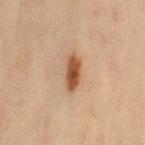Notes:
* biopsy status: catalogued during a skin exam; not biopsied
* image: ~15 mm tile from a whole-body skin photo
* patient: female, approximately 45 years of age
* site: the left thigh